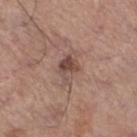Clinical impression:
This lesion was catalogued during total-body skin photography and was not selected for biopsy.
Image and clinical context:
The lesion's longest dimension is about 3.5 mm. The lesion is on the left lower leg. A male subject aged 68–72. Cropped from a total-body skin-imaging series; the visible field is about 15 mm. The lesion-visualizer software estimated a lesion color around L≈48 a*≈18 b*≈23 in CIELAB and a normalized border contrast of about 7.5. The software also gave a border-irregularity rating of about 3.5/10 and a peripheral color-asymmetry measure near 2.5. The tile uses white-light illumination.